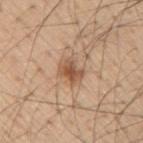Q: What lighting was used for the tile?
A: white-light
Q: Lesion location?
A: the right upper arm
Q: How large is the lesion?
A: ~3 mm (longest diameter)
Q: What kind of image is this?
A: 15 mm crop, total-body photography
Q: What are the patient's age and sex?
A: male, aged approximately 55